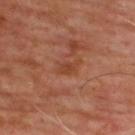No biopsy was performed on this lesion — it was imaged during a full skin examination and was not determined to be concerning. A male subject, roughly 65 years of age. The lesion-visualizer software estimated a footprint of about 3 mm², an outline eccentricity of about 0.85 (0 = round, 1 = elongated), and a symmetry-axis asymmetry near 0.4. It also reported a lesion color around L≈41 a*≈25 b*≈32 in CIELAB, a lesion–skin lightness drop of about 6, and a lesion-to-skin contrast of about 6 (normalized; higher = more distinct). And it measured a lesion-detection confidence of about 100/100. A 15 mm close-up tile from a total-body photography series done for melanoma screening. The lesion is located on the upper back.